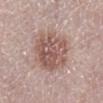| feature | finding |
|---|---|
| notes | total-body-photography surveillance lesion; no biopsy |
| automated metrics | an average lesion color of about L≈55 a*≈19 b*≈23 (CIELAB) and about 11 CIELAB-L* units darker than the surrounding skin; border irregularity of about 2 on a 0–10 scale, a color-variation rating of about 5.5/10, and a peripheral color-asymmetry measure near 2; an automated nevus-likeness rating near 20 out of 100 and a detector confidence of about 100 out of 100 that the crop contains a lesion |
| image | total-body-photography crop, ~15 mm field of view |
| lighting | white-light |
| size | ~6 mm (longest diameter) |
| subject | female, aged 63 to 67 |
| anatomic site | the right lower leg |